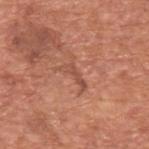Q: Was this lesion biopsied?
A: catalogued during a skin exam; not biopsied
Q: Patient demographics?
A: male, aged approximately 65
Q: What is the anatomic site?
A: the upper back
Q: What is the imaging modality?
A: total-body-photography crop, ~15 mm field of view
Q: What is the lesion's diameter?
A: ~3.5 mm (longest diameter)
Q: What did automated image analysis measure?
A: a footprint of about 3 mm² and an outline eccentricity of about 0.95 (0 = round, 1 = elongated); an average lesion color of about L≈50 a*≈25 b*≈30 (CIELAB), a lesion–skin lightness drop of about 8, and a normalized lesion–skin contrast near 6